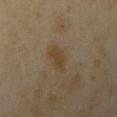Impression:
Captured during whole-body skin photography for melanoma surveillance; the lesion was not biopsied.
Image and clinical context:
A female patient about 35 years old. The lesion is on the right upper arm. A roughly 15 mm field-of-view crop from a total-body skin photograph.